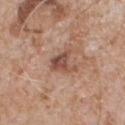An algorithmic analysis of the crop reported an area of roughly 5.5 mm², an outline eccentricity of about 0.55 (0 = round, 1 = elongated), and a symmetry-axis asymmetry near 0.55. Imaged with white-light lighting. The lesion is located on the chest. A male subject, aged 63–67. A roughly 15 mm field-of-view crop from a total-body skin photograph. Measured at roughly 3 mm in maximum diameter.